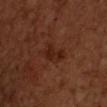Clinical impression:
No biopsy was performed on this lesion — it was imaged during a full skin examination and was not determined to be concerning.